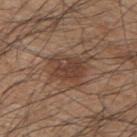The lesion was tiled from a total-body skin photograph and was not biopsied.
Imaged with white-light lighting.
A male patient aged around 45.
The recorded lesion diameter is about 4 mm.
The lesion is on the upper back.
This image is a 15 mm lesion crop taken from a total-body photograph.